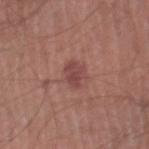* follow-up: catalogued during a skin exam; not biopsied
* tile lighting: white-light illumination
* acquisition: 15 mm crop, total-body photography
* patient: male, aged around 45
* anatomic site: the left thigh